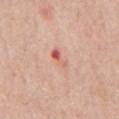Q: How was this image acquired?
A: 15 mm crop, total-body photography
Q: Patient demographics?
A: male, aged approximately 60
Q: Automated lesion metrics?
A: a footprint of about 5 mm², an outline eccentricity of about 0.85 (0 = round, 1 = elongated), and a shape-asymmetry score of about 0.25 (0 = symmetric); a border-irregularity rating of about 2.5/10, a within-lesion color-variation index near 10/10, and peripheral color asymmetry of about 8; a nevus-likeness score of about 0/100 and lesion-presence confidence of about 100/100
Q: Lesion location?
A: the chest
Q: Illumination type?
A: white-light
Q: How large is the lesion?
A: ≈3 mm